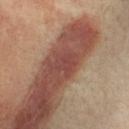Imaged during a routine full-body skin examination; the lesion was not biopsied and no histopathology is available. Longest diameter approximately 3 mm. A 15 mm crop from a total-body photograph taken for skin-cancer surveillance. On the head or neck. Imaged with cross-polarized lighting. A female patient in their mid- to late 70s.